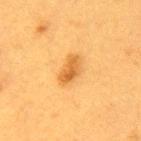Impression: The lesion was photographed on a routine skin check and not biopsied; there is no pathology result. Background: This is a cross-polarized tile. A female subject aged approximately 40. On the back. A lesion tile, about 15 mm wide, cut from a 3D total-body photograph. Automated tile analysis of the lesion measured a lesion area of about 6 mm² and two-axis asymmetry of about 0.2. The analysis additionally found a mean CIELAB color near L≈52 a*≈22 b*≈44 and a lesion–skin lightness drop of about 10. The software also gave a border-irregularity index near 2/10, internal color variation of about 4 on a 0–10 scale, and a peripheral color-asymmetry measure near 1.5. The analysis additionally found an automated nevus-likeness rating near 100 out of 100 and a lesion-detection confidence of about 100/100. About 3.5 mm across.